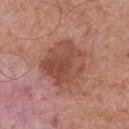{"biopsy_status": "not biopsied; imaged during a skin examination", "image": {"source": "total-body photography crop", "field_of_view_mm": 15}, "site": "front of the torso", "patient": {"sex": "male", "age_approx": 70}}